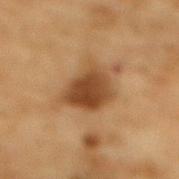automated metrics = a footprint of about 13 mm² and a shape eccentricity near 0.7; a lesion color around L≈39 a*≈18 b*≈32 in CIELAB, a lesion–skin lightness drop of about 12, and a normalized border contrast of about 10; a border-irregularity index near 3/10, a within-lesion color-variation index near 4.5/10, and a peripheral color-asymmetry measure near 1.5 | illumination = cross-polarized illumination | anatomic site = the mid back | imaging modality = ~15 mm crop, total-body skin-cancer survey | patient = male, roughly 85 years of age or older.